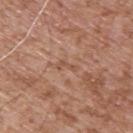Impression:
Recorded during total-body skin imaging; not selected for excision or biopsy.
Background:
About 2.5 mm across. Cropped from a total-body skin-imaging series; the visible field is about 15 mm. On the upper back. The tile uses white-light illumination. A male patient, aged 53 to 57. The total-body-photography lesion software estimated a lesion area of about 2 mm², an outline eccentricity of about 0.9 (0 = round, 1 = elongated), and a symmetry-axis asymmetry near 0.7. The software also gave a lesion–skin lightness drop of about 6. And it measured a border-irregularity rating of about 7.5/10, internal color variation of about 0 on a 0–10 scale, and a peripheral color-asymmetry measure near 0. It also reported a nevus-likeness score of about 0/100 and a lesion-detection confidence of about 60/100.